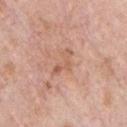Part of a total-body skin-imaging series; this lesion was reviewed on a skin check and was not flagged for biopsy.
A 15 mm close-up tile from a total-body photography series done for melanoma screening.
Automated tile analysis of the lesion measured a lesion area of about 4 mm², an eccentricity of roughly 0.85, and a symmetry-axis asymmetry near 0.65. The analysis additionally found a lesion color around L≈59 a*≈22 b*≈31 in CIELAB, roughly 8 lightness units darker than nearby skin, and a lesion-to-skin contrast of about 5.5 (normalized; higher = more distinct).
About 3.5 mm across.
A male patient in their 70s.
Imaged with white-light lighting.
The lesion is on the chest.